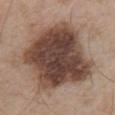This lesion was catalogued during total-body skin photography and was not selected for biopsy. Imaged with white-light lighting. A 15 mm close-up tile from a total-body photography series done for melanoma screening. Located on the right upper arm. About 9.5 mm across. A male subject approximately 55 years of age.